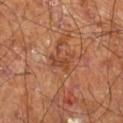workup = catalogued during a skin exam; not biopsied | image = total-body-photography crop, ~15 mm field of view | subject = male, aged around 60 | tile lighting = cross-polarized illumination | site = the right leg | lesion size = about 3.5 mm.